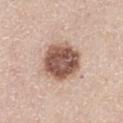Imaged during a routine full-body skin examination; the lesion was not biopsied and no histopathology is available.
Approximately 5 mm at its widest.
A 15 mm close-up extracted from a 3D total-body photography capture.
The lesion is on the left thigh.
A female patient roughly 45 years of age.
This is a white-light tile.